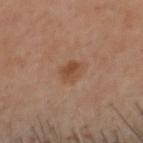biopsy status = total-body-photography surveillance lesion; no biopsy | subject = male, aged around 50 | illumination = cross-polarized illumination | site = the head or neck | acquisition = ~15 mm crop, total-body skin-cancer survey | image-analysis metrics = roughly 7 lightness units darker than nearby skin and a normalized border contrast of about 7; a border-irregularity rating of about 2/10, a within-lesion color-variation index near 3/10, and peripheral color asymmetry of about 1.